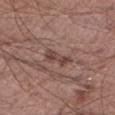Impression: Imaged during a routine full-body skin examination; the lesion was not biopsied and no histopathology is available. Context: A 15 mm crop from a total-body photograph taken for skin-cancer surveillance. From the right lower leg. A male patient in their mid-40s. Measured at roughly 3.5 mm in maximum diameter. Captured under white-light illumination.